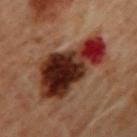{
  "biopsy_status": "not biopsied; imaged during a skin examination",
  "image": {
    "source": "total-body photography crop",
    "field_of_view_mm": 15
  },
  "automated_metrics": {
    "color_variation_0_10": 10.0,
    "nevus_likeness_0_100": 0
  },
  "site": "upper back",
  "patient": {
    "sex": "female",
    "age_approx": 60
  },
  "lighting": "cross-polarized"
}